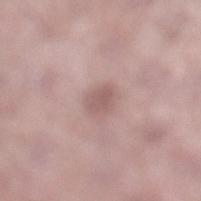No biopsy was performed on this lesion — it was imaged during a full skin examination and was not determined to be concerning. About 2.5 mm across. From the left lower leg. A 15 mm close-up tile from a total-body photography series done for melanoma screening. Captured under white-light illumination. A male patient aged approximately 55.